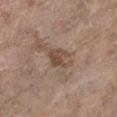The lesion was tiled from a total-body skin photograph and was not biopsied.
The lesion-visualizer software estimated a lesion color around L≈47 a*≈16 b*≈26 in CIELAB, roughly 9 lightness units darker than nearby skin, and a lesion-to-skin contrast of about 7 (normalized; higher = more distinct). The analysis additionally found a classifier nevus-likeness of about 20/100 and a lesion-detection confidence of about 100/100.
A female subject, aged 83 or over.
From the left lower leg.
About 3 mm across.
A 15 mm close-up tile from a total-body photography series done for melanoma screening.
Imaged with white-light lighting.